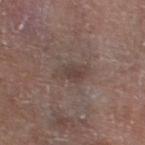Findings:
* biopsy status: no biopsy performed (imaged during a skin exam)
* location: the right lower leg
* tile lighting: white-light
* image: ~15 mm tile from a whole-body skin photo
* subject: male, in their mid-60s
* lesion diameter: ~4 mm (longest diameter)